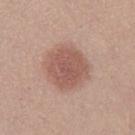Impression:
No biopsy was performed on this lesion — it was imaged during a full skin examination and was not determined to be concerning.
Acquisition and patient details:
Cropped from a total-body skin-imaging series; the visible field is about 15 mm. Imaged with white-light lighting. The patient is a female approximately 20 years of age. From the left thigh. About 4.5 mm across.